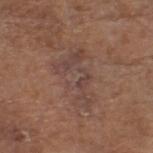Notes:
- anatomic site: the head or neck
- image: 15 mm crop, total-body photography
- image-analysis metrics: an eccentricity of roughly 0.85 and a symmetry-axis asymmetry near 0.5; an automated nevus-likeness rating near 0 out of 100 and lesion-presence confidence of about 65/100
- patient: male, about 80 years old
- illumination: white-light